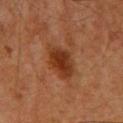| field | value |
|---|---|
| subject | male, aged 58 to 62 |
| site | the upper back |
| image | ~15 mm tile from a whole-body skin photo |